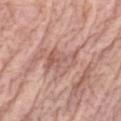Q: Was a biopsy performed?
A: imaged on a skin check; not biopsied